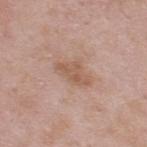Case summary:
• notes — no biopsy performed (imaged during a skin exam)
• lesion diameter — about 4 mm
• automated metrics — an eccentricity of roughly 0.85 and a symmetry-axis asymmetry near 0.3; a lesion color around L≈56 a*≈20 b*≈29 in CIELAB, roughly 8 lightness units darker than nearby skin, and a lesion-to-skin contrast of about 6.5 (normalized; higher = more distinct); a color-variation rating of about 2/10 and radial color variation of about 1; a classifier nevus-likeness of about 0/100 and a lesion-detection confidence of about 100/100
• subject — male, roughly 55 years of age
• tile lighting — white-light illumination
• image — ~15 mm crop, total-body skin-cancer survey
• body site — the upper back